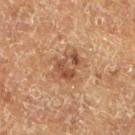| key | value |
|---|---|
| image source | ~15 mm crop, total-body skin-cancer survey |
| patient | male, in their mid-70s |
| anatomic site | the left lower leg |